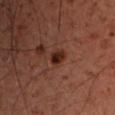{
  "image": {
    "source": "total-body photography crop",
    "field_of_view_mm": 15
  },
  "patient": {
    "sex": "male",
    "age_approx": 50
  },
  "site": "left upper arm"
}